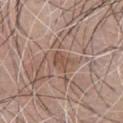Context:
About 2.5 mm across. An algorithmic analysis of the crop reported a lesion area of about 4 mm² and a symmetry-axis asymmetry near 0.3. The analysis additionally found a normalized lesion–skin contrast near 6.5. A 15 mm crop from a total-body photograph taken for skin-cancer surveillance. A male patient in their mid- to late 70s. Captured under white-light illumination. From the chest.